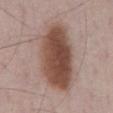Clinical impression: Imaged during a routine full-body skin examination; the lesion was not biopsied and no histopathology is available. Acquisition and patient details: Located on the abdomen. A male subject, roughly 70 years of age. A roughly 15 mm field-of-view crop from a total-body skin photograph.